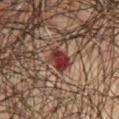Q: Was a biopsy performed?
A: catalogued during a skin exam; not biopsied
Q: What is the lesion's diameter?
A: about 3 mm
Q: What are the patient's age and sex?
A: male, aged approximately 75
Q: What lighting was used for the tile?
A: cross-polarized
Q: How was this image acquired?
A: 15 mm crop, total-body photography
Q: Automated lesion metrics?
A: a peripheral color-asymmetry measure near 2; a detector confidence of about 100 out of 100 that the crop contains a lesion
Q: Where on the body is the lesion?
A: the chest